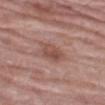The lesion's longest dimension is about 3.5 mm.
This image is a 15 mm lesion crop taken from a total-body photograph.
Captured under white-light illumination.
The lesion is located on the left thigh.
The patient is a female about 75 years old.